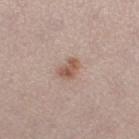biopsy status=no biopsy performed (imaged during a skin exam) | image=15 mm crop, total-body photography | location=the left thigh | lesion diameter=about 3 mm | tile lighting=white-light illumination | subject=female, about 25 years old.